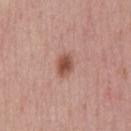<lesion>
<biopsy_status>not biopsied; imaged during a skin examination</biopsy_status>
<site>chest</site>
<lesion_size>
  <long_diameter_mm_approx>2.5</long_diameter_mm_approx>
</lesion_size>
<patient>
  <sex>male</sex>
  <age_approx>45</age_approx>
</patient>
<image>
  <source>total-body photography crop</source>
  <field_of_view_mm>15</field_of_view_mm>
</image>
</lesion>